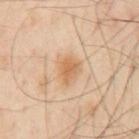Imaged during a routine full-body skin examination; the lesion was not biopsied and no histopathology is available.